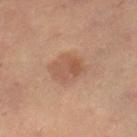Findings:
• workup: no biopsy performed (imaged during a skin exam)
• size: ≈4 mm
• body site: the right thigh
• patient: female, aged around 35
• acquisition: total-body-photography crop, ~15 mm field of view
• tile lighting: cross-polarized illumination